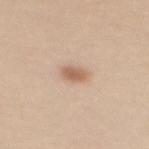Recorded during total-body skin imaging; not selected for excision or biopsy. A female patient, aged 28–32. Longest diameter approximately 2.5 mm. A 15 mm close-up extracted from a 3D total-body photography capture. On the mid back. Imaged with white-light lighting. The total-body-photography lesion software estimated a lesion area of about 3.5 mm², a shape eccentricity near 0.7, and two-axis asymmetry of about 0.3. The software also gave a normalized lesion–skin contrast near 7.5.